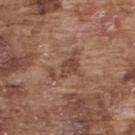Clinical impression: Recorded during total-body skin imaging; not selected for excision or biopsy. Image and clinical context: Located on the upper back. A male patient, in their mid- to late 70s. Automated image analysis of the tile measured a footprint of about 6 mm², an outline eccentricity of about 0.85 (0 = round, 1 = elongated), and a symmetry-axis asymmetry near 0.7. And it measured a border-irregularity rating of about 9/10 and a peripheral color-asymmetry measure near 0.5. A roughly 15 mm field-of-view crop from a total-body skin photograph.